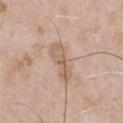follow-up — no biopsy performed (imaged during a skin exam)
patient — male, aged 48–52
diameter — ≈5 mm
site — the chest
illumination — white-light illumination
acquisition — 15 mm crop, total-body photography